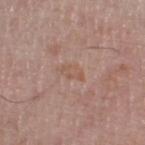The lesion was tiled from a total-body skin photograph and was not biopsied. On the right thigh. This is a white-light tile. A 15 mm close-up tile from a total-body photography series done for melanoma screening. A male patient roughly 70 years of age.